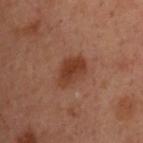| key | value |
|---|---|
| notes | no biopsy performed (imaged during a skin exam) |
| image-analysis metrics | a lesion area of about 7.5 mm² and two-axis asymmetry of about 0.3; a border-irregularity rating of about 2.5/10 and radial color variation of about 1; an automated nevus-likeness rating near 90 out of 100 and a lesion-detection confidence of about 100/100 |
| patient | female, in their mid- to late 50s |
| site | the upper back |
| lesion size | ≈4 mm |
| lighting | cross-polarized illumination |
| acquisition | 15 mm crop, total-body photography |